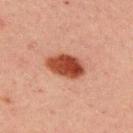{"biopsy_status": "not biopsied; imaged during a skin examination", "image": {"source": "total-body photography crop", "field_of_view_mm": 15}, "lesion_size": {"long_diameter_mm_approx": 5.0}, "site": "back", "lighting": "cross-polarized", "patient": {"sex": "male", "age_approx": 30}}